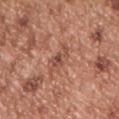Captured during whole-body skin photography for melanoma surveillance; the lesion was not biopsied.
A male subject, aged approximately 55.
A 15 mm close-up extracted from a 3D total-body photography capture.
From the chest.